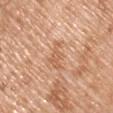{"biopsy_status": "not biopsied; imaged during a skin examination", "site": "left upper arm", "image": {"source": "total-body photography crop", "field_of_view_mm": 15}, "lesion_size": {"long_diameter_mm_approx": 3.5}, "patient": {"sex": "male", "age_approx": 50}}